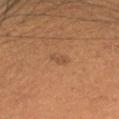Clinical summary:
Automated tile analysis of the lesion measured a border-irregularity index near 3.5/10, a within-lesion color-variation index near 0/10, and radial color variation of about 0. It also reported a detector confidence of about 100 out of 100 that the crop contains a lesion. The patient is a female aged 28–32. The lesion is on the head or neck. The tile uses cross-polarized illumination. The recorded lesion diameter is about 2 mm. This image is a 15 mm lesion crop taken from a total-body photograph.